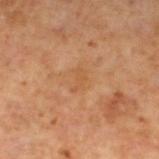Case summary:
• body site · the leg
• tile lighting · cross-polarized
• subject · male, aged 58 to 62
• automated lesion analysis · an outline eccentricity of about 0.85 (0 = round, 1 = elongated) and a shape-asymmetry score of about 0.55 (0 = symmetric); an average lesion color of about L≈51 a*≈21 b*≈37 (CIELAB) and a lesion–skin lightness drop of about 5
• image · ~15 mm tile from a whole-body skin photo
• lesion size · ~2.5 mm (longest diameter)